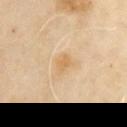Q: Is there a histopathology result?
A: no biopsy performed (imaged during a skin exam)
Q: Where on the body is the lesion?
A: the right upper arm
Q: Who is the patient?
A: male, in their mid- to late 60s
Q: What kind of image is this?
A: ~15 mm tile from a whole-body skin photo
Q: Automated lesion metrics?
A: an eccentricity of roughly 0.65 and two-axis asymmetry of about 0.35; a border-irregularity index near 3/10, a color-variation rating of about 1/10, and a peripheral color-asymmetry measure near 0.5
Q: How large is the lesion?
A: about 2.5 mm
Q: Illumination type?
A: cross-polarized illumination